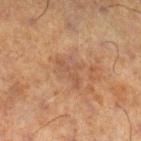The lesion was photographed on a routine skin check and not biopsied; there is no pathology result. The subject is a male approximately 70 years of age. Located on the right lower leg. This image is a 15 mm lesion crop taken from a total-body photograph.